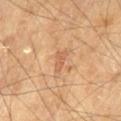follow-up: no biopsy performed (imaged during a skin exam)
patient: male, aged approximately 70
acquisition: 15 mm crop, total-body photography
anatomic site: the left thigh